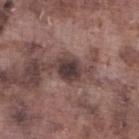Notes:
– workup — no biopsy performed (imaged during a skin exam)
– imaging modality — ~15 mm crop, total-body skin-cancer survey
– illumination — white-light
– location — the left lower leg
– patient — male, approximately 75 years of age